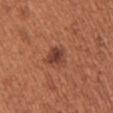image source — ~15 mm tile from a whole-body skin photo | subject — female, approximately 65 years of age | image-analysis metrics — a footprint of about 5.5 mm² and an outline eccentricity of about 0.6 (0 = round, 1 = elongated); an average lesion color of about L≈41 a*≈25 b*≈28 (CIELAB); border irregularity of about 2 on a 0–10 scale, internal color variation of about 4 on a 0–10 scale, and a peripheral color-asymmetry measure near 1; a nevus-likeness score of about 70/100 and lesion-presence confidence of about 100/100 | illumination — white-light | body site — the chest | size — ~3 mm (longest diameter).